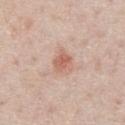<tbp_lesion>
  <biopsy_status>not biopsied; imaged during a skin examination</biopsy_status>
  <automated_metrics>
    <shape_asymmetry>0.25</shape_asymmetry>
    <cielab_L>62</cielab_L>
    <cielab_a>22</cielab_a>
    <cielab_b>29</cielab_b>
    <vs_skin_darker_L>10.0</vs_skin_darker_L>
    <vs_skin_contrast_norm>6.5</vs_skin_contrast_norm>
    <lesion_detection_confidence_0_100>100</lesion_detection_confidence_0_100>
  </automated_metrics>
  <lighting>white-light</lighting>
  <site>chest</site>
  <image>
    <source>total-body photography crop</source>
    <field_of_view_mm>15</field_of_view_mm>
  </image>
  <patient>
    <sex>male</sex>
    <age_approx>60</age_approx>
  </patient>
</tbp_lesion>